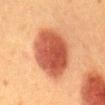• notes · total-body-photography surveillance lesion; no biopsy
• patient · female, roughly 50 years of age
• lesion size · ~7 mm (longest diameter)
• image source · ~15 mm crop, total-body skin-cancer survey
• tile lighting · cross-polarized illumination
• body site · the mid back